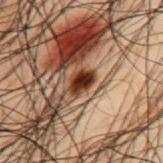Impression:
No biopsy was performed on this lesion — it was imaged during a full skin examination and was not determined to be concerning.
Background:
A close-up tile cropped from a whole-body skin photograph, about 15 mm across. A male subject, about 50 years old. The lesion is on the mid back. Imaged with cross-polarized lighting. The recorded lesion diameter is about 3 mm. The lesion-visualizer software estimated a lesion area of about 4.5 mm², an outline eccentricity of about 0.8 (0 = round, 1 = elongated), and two-axis asymmetry of about 0.25. And it measured internal color variation of about 5 on a 0–10 scale.